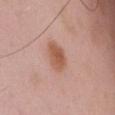Assessment: The lesion was tiled from a total-body skin photograph and was not biopsied. Background: An algorithmic analysis of the crop reported a color-variation rating of about 3/10 and a peripheral color-asymmetry measure near 1. The analysis additionally found a nevus-likeness score of about 100/100 and a lesion-detection confidence of about 100/100. A male subject aged around 55. Approximately 4 mm at its widest. From the chest. A 15 mm close-up tile from a total-body photography series done for melanoma screening. This is a white-light tile.